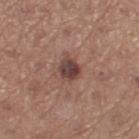Q: Is there a histopathology result?
A: imaged on a skin check; not biopsied
Q: Patient demographics?
A: female, in their mid- to late 60s
Q: What is the lesion's diameter?
A: ≈3 mm
Q: Illumination type?
A: white-light
Q: What is the anatomic site?
A: the leg
Q: What did automated image analysis measure?
A: an area of roughly 5.5 mm², a shape eccentricity near 0.55, and two-axis asymmetry of about 0.2; an average lesion color of about L≈42 a*≈19 b*≈22 (CIELAB), about 12 CIELAB-L* units darker than the surrounding skin, and a normalized lesion–skin contrast near 10; a within-lesion color-variation index near 5/10 and peripheral color asymmetry of about 1.5
Q: What kind of image is this?
A: total-body-photography crop, ~15 mm field of view